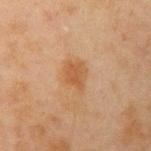Case summary:
– automated metrics · a lesion area of about 6 mm² and a shape eccentricity near 0.65; a border-irregularity rating of about 3.5/10, a within-lesion color-variation index near 2.5/10, and radial color variation of about 1; a nevus-likeness score of about 55/100 and lesion-presence confidence of about 100/100
– patient · male, in their mid-40s
– image · ~15 mm tile from a whole-body skin photo
– size · ≈3.5 mm
– site · the right upper arm